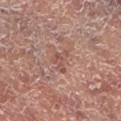Q: Was this lesion biopsied?
A: imaged on a skin check; not biopsied
Q: What are the patient's age and sex?
A: male, aged 53 to 57
Q: How was this image acquired?
A: 15 mm crop, total-body photography
Q: Where on the body is the lesion?
A: the left lower leg